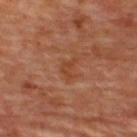No biopsy was performed on this lesion — it was imaged during a full skin examination and was not determined to be concerning.
A female subject aged 43–47.
The tile uses cross-polarized illumination.
Longest diameter approximately 2.5 mm.
A 15 mm close-up tile from a total-body photography series done for melanoma screening.
On the upper back.